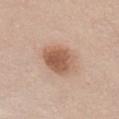Recorded during total-body skin imaging; not selected for excision or biopsy. A female patient in their mid- to late 60s. From the front of the torso. Cropped from a whole-body photographic skin survey; the tile spans about 15 mm. The lesion's longest dimension is about 4.5 mm. This is a white-light tile.